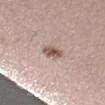Case summary:
* workup — imaged on a skin check; not biopsied
* diameter — ~3 mm (longest diameter)
* patient — female, approximately 25 years of age
* image source — ~15 mm crop, total-body skin-cancer survey
* lighting — white-light
* anatomic site — the arm
* TBP lesion metrics — a shape-asymmetry score of about 0.25 (0 = symmetric); an average lesion color of about L≈55 a*≈18 b*≈24 (CIELAB), about 13 CIELAB-L* units darker than the surrounding skin, and a normalized lesion–skin contrast near 9; a nevus-likeness score of about 90/100 and a detector confidence of about 100 out of 100 that the crop contains a lesion